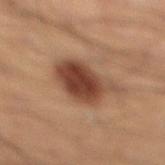Context: Captured under cross-polarized illumination. Automated image analysis of the tile measured a lesion area of about 17 mm², a shape eccentricity near 0.7, and a shape-asymmetry score of about 0.25 (0 = symmetric). The analysis additionally found an average lesion color of about L≈38 a*≈19 b*≈27 (CIELAB), a lesion–skin lightness drop of about 13, and a normalized lesion–skin contrast near 11. The analysis additionally found internal color variation of about 5 on a 0–10 scale and radial color variation of about 1.5. A 15 mm crop from a total-body photograph taken for skin-cancer surveillance. The patient is a male in their 40s. The lesion is on the right lower leg.